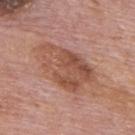The lesion was tiled from a total-body skin photograph and was not biopsied. The tile uses white-light illumination. On the upper back. About 7 mm across. A 15 mm close-up tile from a total-body photography series done for melanoma screening. A male patient, aged 73–77.